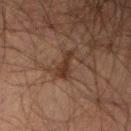notes: no biopsy performed (imaged during a skin exam)
automated lesion analysis: a footprint of about 4.5 mm², an eccentricity of roughly 0.8, and two-axis asymmetry of about 0.4; roughly 8 lightness units darker than nearby skin and a normalized border contrast of about 8.5; a lesion-detection confidence of about 100/100
diameter: ≈3 mm
location: the left forearm
acquisition: ~15 mm tile from a whole-body skin photo
subject: male, roughly 50 years of age